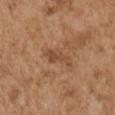Imaged during a routine full-body skin examination; the lesion was not biopsied and no histopathology is available.